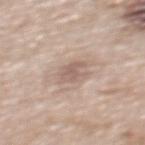Findings:
• follow-up — catalogued during a skin exam; not biopsied
• subject — male, aged around 70
• body site — the back
• acquisition — ~15 mm tile from a whole-body skin photo
• tile lighting — white-light
• lesion diameter — about 3 mm
• automated lesion analysis — a mean CIELAB color near L≈60 a*≈16 b*≈24 and a normalized lesion–skin contrast near 6; border irregularity of about 3.5 on a 0–10 scale, internal color variation of about 2 on a 0–10 scale, and a peripheral color-asymmetry measure near 0.5; a nevus-likeness score of about 0/100 and a detector confidence of about 100 out of 100 that the crop contains a lesion